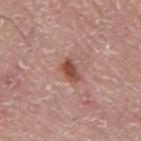<case>
<site>mid back</site>
<patient>
  <sex>male</sex>
  <age_approx>75</age_approx>
</patient>
<lesion_size>
  <long_diameter_mm_approx>3.0</long_diameter_mm_approx>
</lesion_size>
<lighting>white-light</lighting>
<image>
  <source>total-body photography crop</source>
  <field_of_view_mm>15</field_of_view_mm>
</image>
</case>